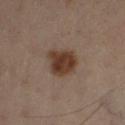Q: Was a biopsy performed?
A: catalogued during a skin exam; not biopsied
Q: Patient demographics?
A: male, aged approximately 55
Q: What is the imaging modality?
A: ~15 mm tile from a whole-body skin photo
Q: Illumination type?
A: cross-polarized
Q: Automated lesion metrics?
A: an average lesion color of about L≈29 a*≈14 b*≈21 (CIELAB), roughly 10 lightness units darker than nearby skin, and a normalized lesion–skin contrast near 10; peripheral color asymmetry of about 1; an automated nevus-likeness rating near 95 out of 100 and a lesion-detection confidence of about 100/100
Q: What is the lesion's diameter?
A: ≈4.5 mm
Q: What is the anatomic site?
A: the left lower leg